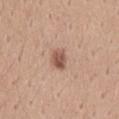The lesion was tiled from a total-body skin photograph and was not biopsied.